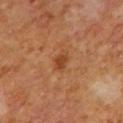notes = total-body-photography surveillance lesion; no biopsy | lesion diameter = ≈2.5 mm | location = the mid back | patient = male, aged 63 to 67 | lighting = cross-polarized | image = ~15 mm crop, total-body skin-cancer survey.